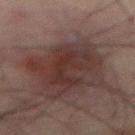Q: Was this lesion biopsied?
A: catalogued during a skin exam; not biopsied
Q: What kind of image is this?
A: total-body-photography crop, ~15 mm field of view
Q: Who is the patient?
A: male, roughly 65 years of age
Q: How was the tile lit?
A: cross-polarized
Q: Lesion size?
A: ≈5.5 mm
Q: What did automated image analysis measure?
A: a lesion area of about 13 mm², an eccentricity of roughly 0.85, and a symmetry-axis asymmetry near 0.4; a lesion–skin lightness drop of about 6 and a normalized lesion–skin contrast near 7; a border-irregularity index near 6/10, internal color variation of about 4 on a 0–10 scale, and radial color variation of about 1.5
Q: What is the anatomic site?
A: the lower back